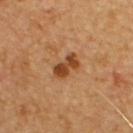Captured during whole-body skin photography for melanoma surveillance; the lesion was not biopsied. From the chest. A male subject, in their mid-50s. Imaged with cross-polarized lighting. Cropped from a whole-body photographic skin survey; the tile spans about 15 mm. The lesion's longest dimension is about 3.5 mm.